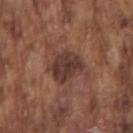Q: Was this lesion biopsied?
A: imaged on a skin check; not biopsied
Q: Where on the body is the lesion?
A: the right upper arm
Q: What is the imaging modality?
A: ~15 mm tile from a whole-body skin photo
Q: How was the tile lit?
A: white-light
Q: Patient demographics?
A: male, in their mid- to late 70s